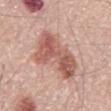{
  "biopsy_status": "not biopsied; imaged during a skin examination"
}